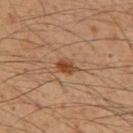The subject is a male aged around 50. This is a cross-polarized tile. On the back. A close-up tile cropped from a whole-body skin photograph, about 15 mm across. The lesion-visualizer software estimated an area of roughly 4 mm², an outline eccentricity of about 0.7 (0 = round, 1 = elongated), and two-axis asymmetry of about 0.25. The analysis additionally found a mean CIELAB color near L≈44 a*≈21 b*≈33, roughly 10 lightness units darker than nearby skin, and a normalized lesion–skin contrast near 8. It also reported a nevus-likeness score of about 95/100 and a detector confidence of about 100 out of 100 that the crop contains a lesion.